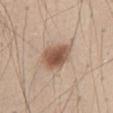This lesion was catalogued during total-body skin photography and was not selected for biopsy.
A region of skin cropped from a whole-body photographic capture, roughly 15 mm wide.
A male subject in their mid- to late 40s.
From the abdomen.